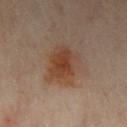The lesion was photographed on a routine skin check and not biopsied; there is no pathology result.
Captured under cross-polarized illumination.
A female subject, aged approximately 60.
A close-up tile cropped from a whole-body skin photograph, about 15 mm across.
The lesion is on the right leg.
The lesion's longest dimension is about 5.5 mm.